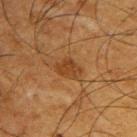The lesion was tiled from a total-body skin photograph and was not biopsied.
The recorded lesion diameter is about 3.5 mm.
A 15 mm close-up extracted from a 3D total-body photography capture.
The lesion-visualizer software estimated a border-irregularity index near 2/10 and a within-lesion color-variation index near 2/10. And it measured an automated nevus-likeness rating near 55 out of 100 and a lesion-detection confidence of about 100/100.
A male subject in their mid- to late 60s.
The lesion is on the back.